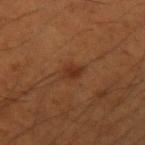Q: What is the imaging modality?
A: ~15 mm crop, total-body skin-cancer survey
Q: What are the patient's age and sex?
A: male, in their mid-50s
Q: How was the tile lit?
A: cross-polarized illumination
Q: What is the anatomic site?
A: the left upper arm
Q: What did automated image analysis measure?
A: an area of roughly 3.5 mm², a shape eccentricity near 0.75, and a symmetry-axis asymmetry near 0.3; a mean CIELAB color near L≈32 a*≈22 b*≈31 and a normalized lesion–skin contrast near 7; an automated nevus-likeness rating near 50 out of 100 and a lesion-detection confidence of about 100/100